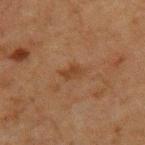<lesion>
<biopsy_status>not biopsied; imaged during a skin examination</biopsy_status>
<lesion_size>
  <long_diameter_mm_approx>3.0</long_diameter_mm_approx>
</lesion_size>
<automated_metrics>
  <nevus_likeness_0_100>5</nevus_likeness_0_100>
  <lesion_detection_confidence_0_100>100</lesion_detection_confidence_0_100>
</automated_metrics>
<patient>
  <sex>male</sex>
  <age_approx>60</age_approx>
</patient>
<lighting>cross-polarized</lighting>
<image>
  <source>total-body photography crop</source>
  <field_of_view_mm>15</field_of_view_mm>
</image>
<site>upper back</site>
</lesion>